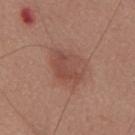Notes:
* biopsy status: total-body-photography surveillance lesion; no biopsy
* patient: male, in their 70s
* body site: the upper back
* acquisition: ~15 mm crop, total-body skin-cancer survey
* tile lighting: white-light